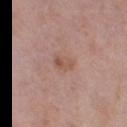This lesion was catalogued during total-body skin photography and was not selected for biopsy.
A close-up tile cropped from a whole-body skin photograph, about 15 mm across.
A female subject, aged approximately 40.
On the right thigh.
Automated tile analysis of the lesion measured a lesion area of about 3.5 mm², an eccentricity of roughly 0.8, and two-axis asymmetry of about 0.3.
Imaged with white-light lighting.
About 2.5 mm across.